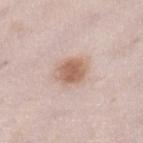Part of a total-body skin-imaging series; this lesion was reviewed on a skin check and was not flagged for biopsy.
A female patient aged 28 to 32.
A roughly 15 mm field-of-view crop from a total-body skin photograph.
The lesion is located on the left lower leg.